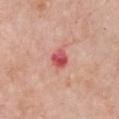Q: What kind of image is this?
A: ~15 mm crop, total-body skin-cancer survey
Q: Who is the patient?
A: female, in their mid-60s
Q: Where on the body is the lesion?
A: the chest